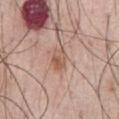Q: Is there a histopathology result?
A: no biopsy performed (imaged during a skin exam)
Q: Automated lesion metrics?
A: an outline eccentricity of about 0.75 (0 = round, 1 = elongated) and a symmetry-axis asymmetry near 0.2; a border-irregularity rating of about 2/10 and a within-lesion color-variation index near 5.5/10; an automated nevus-likeness rating near 40 out of 100 and a lesion-detection confidence of about 100/100
Q: What kind of image is this?
A: 15 mm crop, total-body photography
Q: What is the anatomic site?
A: the chest
Q: Lesion size?
A: ≈3 mm
Q: How was the tile lit?
A: white-light
Q: What are the patient's age and sex?
A: male, approximately 70 years of age